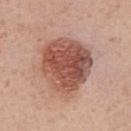Clinical summary:
On the abdomen. Automated image analysis of the tile measured border irregularity of about 2 on a 0–10 scale. This is a white-light tile. A male patient in their mid- to late 50s. Cropped from a whole-body photographic skin survey; the tile spans about 15 mm.